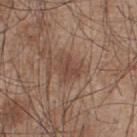notes = no biopsy performed (imaged during a skin exam)
tile lighting = white-light
diameter = about 3 mm
image source = 15 mm crop, total-body photography
location = the upper back
subject = male, aged 43–47